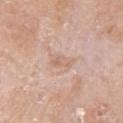follow-up=catalogued during a skin exam; not biopsied
subject=female, roughly 75 years of age
lesion diameter=~3 mm (longest diameter)
image-analysis metrics=an eccentricity of roughly 0.8 and two-axis asymmetry of about 0.3; a nevus-likeness score of about 0/100 and lesion-presence confidence of about 100/100
anatomic site=the arm
image=15 mm crop, total-body photography
illumination=white-light illumination